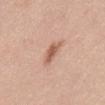<case>
<biopsy_status>not biopsied; imaged during a skin examination</biopsy_status>
<lesion_size>
  <long_diameter_mm_approx>3.5</long_diameter_mm_approx>
</lesion_size>
<patient>
  <sex>male</sex>
  <age_approx>30</age_approx>
</patient>
<site>abdomen</site>
<image>
  <source>total-body photography crop</source>
  <field_of_view_mm>15</field_of_view_mm>
</image>
<lighting>white-light</lighting>
</case>